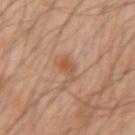Notes:
• workup: imaged on a skin check; not biopsied
• diameter: ~3.5 mm (longest diameter)
• site: the right upper arm
• image source: ~15 mm tile from a whole-body skin photo
• automated lesion analysis: a shape eccentricity near 0.85; border irregularity of about 4 on a 0–10 scale, a color-variation rating of about 2.5/10, and peripheral color asymmetry of about 1; an automated nevus-likeness rating near 65 out of 100 and lesion-presence confidence of about 100/100
• patient: male, aged 43 to 47